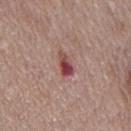On the back.
A 15 mm close-up extracted from a 3D total-body photography capture.
A male patient, in their 70s.